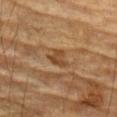Part of a total-body skin-imaging series; this lesion was reviewed on a skin check and was not flagged for biopsy. A region of skin cropped from a whole-body photographic capture, roughly 15 mm wide. A male subject approximately 85 years of age or older. The lesion-visualizer software estimated an outline eccentricity of about 0.6 (0 = round, 1 = elongated) and a symmetry-axis asymmetry near 0.45. And it measured a within-lesion color-variation index near 2.5/10 and peripheral color asymmetry of about 1. From the left forearm. The tile uses cross-polarized illumination.